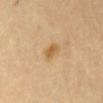No biopsy was performed on this lesion — it was imaged during a full skin examination and was not determined to be concerning. The lesion is on the abdomen. A close-up tile cropped from a whole-body skin photograph, about 15 mm across. Approximately 2.5 mm at its widest. The tile uses cross-polarized illumination. A female subject in their mid- to late 60s. Automated tile analysis of the lesion measured an area of roughly 3 mm², an eccentricity of roughly 0.8, and two-axis asymmetry of about 0.3. The software also gave an average lesion color of about L≈61 a*≈18 b*≈42 (CIELAB), roughly 9 lightness units darker than nearby skin, and a normalized lesion–skin contrast near 7. The software also gave a border-irregularity rating of about 2.5/10, a color-variation rating of about 2.5/10, and peripheral color asymmetry of about 1. The analysis additionally found an automated nevus-likeness rating near 75 out of 100 and a detector confidence of about 100 out of 100 that the crop contains a lesion.